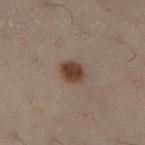Captured during whole-body skin photography for melanoma surveillance; the lesion was not biopsied. Captured under cross-polarized illumination. The subject is a female approximately 30 years of age. From the leg. A 15 mm crop from a total-body photograph taken for skin-cancer surveillance. The total-body-photography lesion software estimated a mean CIELAB color near L≈31 a*≈14 b*≈21, about 10 CIELAB-L* units darker than the surrounding skin, and a normalized border contrast of about 10.5. It also reported a border-irregularity rating of about 1.5/10 and peripheral color asymmetry of about 0.5. The analysis additionally found a classifier nevus-likeness of about 100/100 and a lesion-detection confidence of about 100/100. Longest diameter approximately 3 mm.